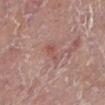Part of a total-body skin-imaging series; this lesion was reviewed on a skin check and was not flagged for biopsy. The total-body-photography lesion software estimated an area of roughly 3.5 mm², an eccentricity of roughly 0.9, and two-axis asymmetry of about 0.45. The analysis additionally found a mean CIELAB color near L≈46 a*≈21 b*≈21 and about 6 CIELAB-L* units darker than the surrounding skin. And it measured a border-irregularity index near 5/10 and a color-variation rating of about 1/10. It also reported lesion-presence confidence of about 100/100. Captured under cross-polarized illumination. The lesion is on the right leg. This image is a 15 mm lesion crop taken from a total-body photograph. The subject is a female approximately 80 years of age. Approximately 3.5 mm at its widest.